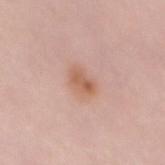The lesion was photographed on a routine skin check and not biopsied; there is no pathology result. A female patient, roughly 50 years of age. A 15 mm close-up extracted from a 3D total-body photography capture. The lesion is on the chest.